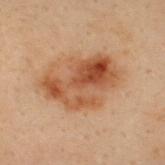The lesion was tiled from a total-body skin photograph and was not biopsied. About 7.5 mm across. On the upper back. Imaged with cross-polarized lighting. A male patient aged approximately 30. A lesion tile, about 15 mm wide, cut from a 3D total-body photograph.